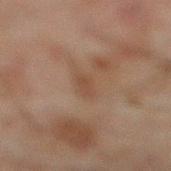Q: Is there a histopathology result?
A: total-body-photography surveillance lesion; no biopsy
Q: Who is the patient?
A: male, aged approximately 45
Q: How large is the lesion?
A: ≈2.5 mm
Q: How was this image acquired?
A: 15 mm crop, total-body photography
Q: How was the tile lit?
A: cross-polarized illumination
Q: What is the anatomic site?
A: the right lower leg